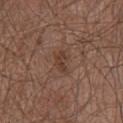<record>
  <biopsy_status>not biopsied; imaged during a skin examination</biopsy_status>
  <patient>
    <sex>male</sex>
    <age_approx>65</age_approx>
  </patient>
  <image>
    <source>total-body photography crop</source>
    <field_of_view_mm>15</field_of_view_mm>
  </image>
  <lighting>white-light</lighting>
  <lesion_size>
    <long_diameter_mm_approx>3.0</long_diameter_mm_approx>
  </lesion_size>
  <site>chest</site>
</record>